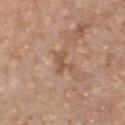biopsy status: no biopsy performed (imaged during a skin exam) | imaging modality: ~15 mm tile from a whole-body skin photo | lesion diameter: about 2.5 mm | lighting: white-light illumination | site: the front of the torso | patient: male, aged around 70.